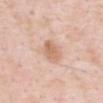Automated tile analysis of the lesion measured a color-variation rating of about 3/10. And it measured a classifier nevus-likeness of about 65/100 and lesion-presence confidence of about 100/100.
A 15 mm close-up tile from a total-body photography series done for melanoma screening.
About 3.5 mm across.
The lesion is located on the chest.
This is a white-light tile.
A male subject aged 48 to 52.